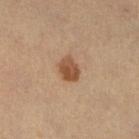workup — imaged on a skin check; not biopsied
illumination — cross-polarized illumination
TBP lesion metrics — a lesion color around L≈49 a*≈20 b*≈33 in CIELAB, a lesion–skin lightness drop of about 12, and a normalized border contrast of about 9; border irregularity of about 2 on a 0–10 scale, internal color variation of about 4 on a 0–10 scale, and peripheral color asymmetry of about 1.5
site — the right lower leg
imaging modality — total-body-photography crop, ~15 mm field of view
lesion diameter — ~3 mm (longest diameter)
patient — female, approximately 45 years of age